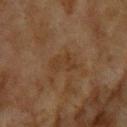Q: Was a biopsy performed?
A: imaged on a skin check; not biopsied
Q: Automated lesion metrics?
A: roughly 5 lightness units darker than nearby skin and a normalized lesion–skin contrast near 5.5
Q: Lesion size?
A: about 3 mm
Q: How was the tile lit?
A: cross-polarized
Q: Patient demographics?
A: female, aged 58–62
Q: What kind of image is this?
A: total-body-photography crop, ~15 mm field of view
Q: What is the anatomic site?
A: the head or neck